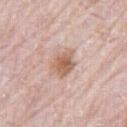No biopsy was performed on this lesion — it was imaged during a full skin examination and was not determined to be concerning.
The lesion is on the right thigh.
A male patient, aged approximately 80.
Imaged with white-light lighting.
The recorded lesion diameter is about 3.5 mm.
The lesion-visualizer software estimated a lesion area of about 6.5 mm², a shape eccentricity near 0.7, and a symmetry-axis asymmetry near 0.3. It also reported a mean CIELAB color near L≈60 a*≈20 b*≈29 and a normalized border contrast of about 8.5. And it measured a border-irregularity index near 3/10 and internal color variation of about 4 on a 0–10 scale.
A 15 mm crop from a total-body photograph taken for skin-cancer surveillance.